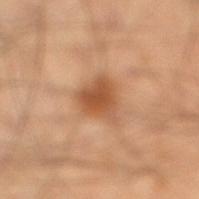{
  "biopsy_status": "not biopsied; imaged during a skin examination",
  "lesion_size": {
    "long_diameter_mm_approx": 3.5
  },
  "patient": {
    "sex": "male",
    "age_approx": 40
  },
  "image": {
    "source": "total-body photography crop",
    "field_of_view_mm": 15
  },
  "site": "leg",
  "automated_metrics": {
    "nevus_likeness_0_100": 85,
    "lesion_detection_confidence_0_100": 100
  },
  "lighting": "cross-polarized"
}